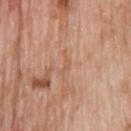The lesion was photographed on a routine skin check and not biopsied; there is no pathology result.